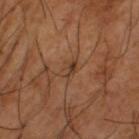notes: no biopsy performed (imaged during a skin exam) | automated metrics: an average lesion color of about L≈39 a*≈19 b*≈30 (CIELAB), a lesion–skin lightness drop of about 8, and a lesion-to-skin contrast of about 6.5 (normalized; higher = more distinct); a classifier nevus-likeness of about 5/100 | patient: male, aged 63–67 | diameter: about 2.5 mm | imaging modality: ~15 mm crop, total-body skin-cancer survey | tile lighting: cross-polarized | location: the left thigh.